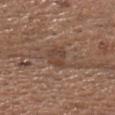Captured during whole-body skin photography for melanoma surveillance; the lesion was not biopsied.
Longest diameter approximately 3 mm.
A male subject, aged 73–77.
Automated tile analysis of the lesion measured a lesion area of about 5.5 mm² and an outline eccentricity of about 0.65 (0 = round, 1 = elongated). The analysis additionally found an average lesion color of about L≈43 a*≈18 b*≈26 (CIELAB), roughly 8 lightness units darker than nearby skin, and a lesion-to-skin contrast of about 6.5 (normalized; higher = more distinct).
Cropped from a total-body skin-imaging series; the visible field is about 15 mm.
Captured under white-light illumination.
On the head or neck.